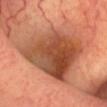Imaged during a routine full-body skin examination; the lesion was not biopsied and no histopathology is available. The recorded lesion diameter is about 8.5 mm. An algorithmic analysis of the crop reported a shape eccentricity near 0.45. It also reported an average lesion color of about L≈48 a*≈25 b*≈34 (CIELAB), roughly 13 lightness units darker than nearby skin, and a lesion-to-skin contrast of about 9 (normalized; higher = more distinct). Captured under cross-polarized illumination. Cropped from a total-body skin-imaging series; the visible field is about 15 mm. A male patient, aged 58 to 62. The lesion is located on the head or neck.